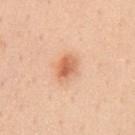Clinical impression:
This lesion was catalogued during total-body skin photography and was not selected for biopsy.
Background:
About 3 mm across. The subject is a male aged 48 to 52. An algorithmic analysis of the crop reported an average lesion color of about L≈65 a*≈26 b*≈37 (CIELAB) and a normalized lesion–skin contrast near 7.5. And it measured a border-irregularity rating of about 1.5/10, a within-lesion color-variation index near 6/10, and a peripheral color-asymmetry measure near 2. It also reported a nevus-likeness score of about 95/100 and a detector confidence of about 100 out of 100 that the crop contains a lesion. The tile uses white-light illumination. A lesion tile, about 15 mm wide, cut from a 3D total-body photograph. Located on the chest.